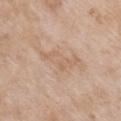No biopsy was performed on this lesion — it was imaged during a full skin examination and was not determined to be concerning. This image is a 15 mm lesion crop taken from a total-body photograph. The lesion is located on the front of the torso. Imaged with white-light lighting. A female patient, in their mid- to late 70s.